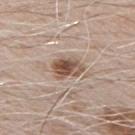* workup: imaged on a skin check; not biopsied
* patient: male, about 70 years old
* body site: the front of the torso
* lighting: white-light
* automated lesion analysis: an area of roughly 7 mm², an outline eccentricity of about 0.75 (0 = round, 1 = elongated), and a shape-asymmetry score of about 0.2 (0 = symmetric); a mean CIELAB color near L≈52 a*≈17 b*≈26, roughly 14 lightness units darker than nearby skin, and a normalized lesion–skin contrast near 10; a border-irregularity index near 2.5/10, internal color variation of about 7.5 on a 0–10 scale, and a peripheral color-asymmetry measure near 2.5; a detector confidence of about 100 out of 100 that the crop contains a lesion
* image: ~15 mm tile from a whole-body skin photo
* lesion size: about 3.5 mm